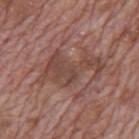Q: Is there a histopathology result?
A: catalogued during a skin exam; not biopsied
Q: Where on the body is the lesion?
A: the mid back
Q: Lesion size?
A: about 7 mm
Q: How was this image acquired?
A: ~15 mm crop, total-body skin-cancer survey
Q: What are the patient's age and sex?
A: male, aged 68–72
Q: Illumination type?
A: white-light illumination